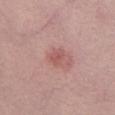Impression: Captured during whole-body skin photography for melanoma surveillance; the lesion was not biopsied. Image and clinical context: Captured under white-light illumination. A female subject aged 63 to 67. Automated tile analysis of the lesion measured a lesion color around L≈54 a*≈26 b*≈23 in CIELAB, a lesion–skin lightness drop of about 8, and a lesion-to-skin contrast of about 5.5 (normalized; higher = more distinct). Located on the abdomen. A 15 mm close-up extracted from a 3D total-body photography capture.